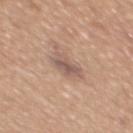Recorded during total-body skin imaging; not selected for excision or biopsy.
From the back.
A male subject, aged around 55.
The tile uses white-light illumination.
A lesion tile, about 15 mm wide, cut from a 3D total-body photograph.
Approximately 4 mm at its widest.